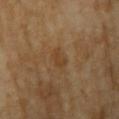Impression:
The lesion was photographed on a routine skin check and not biopsied; there is no pathology result.
Acquisition and patient details:
On the left upper arm. Cropped from a total-body skin-imaging series; the visible field is about 15 mm. Automated image analysis of the tile measured a lesion area of about 3 mm², a shape eccentricity near 0.85, and two-axis asymmetry of about 0.4. And it measured a lesion–skin lightness drop of about 6. Approximately 2.5 mm at its widest. The subject is a female roughly 70 years of age. The tile uses cross-polarized illumination.